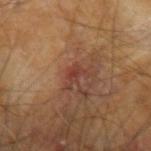Captured during whole-body skin photography for melanoma surveillance; the lesion was not biopsied.
A male patient, aged 58 to 62.
Located on the left arm.
A lesion tile, about 15 mm wide, cut from a 3D total-body photograph.
Measured at roughly 3 mm in maximum diameter.